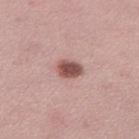Impression:
Part of a total-body skin-imaging series; this lesion was reviewed on a skin check and was not flagged for biopsy.
Image and clinical context:
An algorithmic analysis of the crop reported an area of roughly 5.5 mm², an eccentricity of roughly 0.7, and a symmetry-axis asymmetry near 0.2. It also reported a border-irregularity index near 1.5/10 and peripheral color asymmetry of about 1. It also reported a classifier nevus-likeness of about 95/100 and a detector confidence of about 100 out of 100 that the crop contains a lesion. The lesion is located on the leg. About 3 mm across. The subject is a female roughly 35 years of age. A region of skin cropped from a whole-body photographic capture, roughly 15 mm wide.